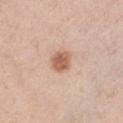{"biopsy_status": "not biopsied; imaged during a skin examination", "site": "chest", "image": {"source": "total-body photography crop", "field_of_view_mm": 15}, "patient": {"sex": "female", "age_approx": 55}, "lighting": "white-light"}